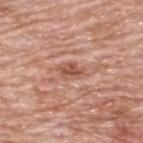Notes:
• biopsy status · imaged on a skin check; not biopsied
• acquisition · ~15 mm tile from a whole-body skin photo
• anatomic site · the upper back
• lighting · white-light illumination
• subject · male, roughly 80 years of age
• lesion size · ~3 mm (longest diameter)